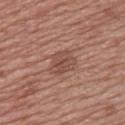The lesion was photographed on a routine skin check and not biopsied; there is no pathology result.
The total-body-photography lesion software estimated a nevus-likeness score of about 0/100 and lesion-presence confidence of about 100/100.
A female patient, approximately 50 years of age.
On the upper back.
A roughly 15 mm field-of-view crop from a total-body skin photograph.
The lesion's longest dimension is about 3.5 mm.